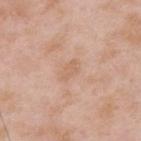{"biopsy_status": "not biopsied; imaged during a skin examination", "site": "upper back", "lighting": "white-light", "lesion_size": {"long_diameter_mm_approx": 2.5}, "image": {"source": "total-body photography crop", "field_of_view_mm": 15}, "patient": {"sex": "male", "age_approx": 55}}